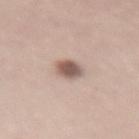workup: catalogued during a skin exam; not biopsied | lesion size: ≈3 mm | patient: female, in their mid- to late 30s | acquisition: 15 mm crop, total-body photography | automated lesion analysis: an area of roughly 5.5 mm² and a shape-asymmetry score of about 0.2 (0 = symmetric); about 15 CIELAB-L* units darker than the surrounding skin and a normalized lesion–skin contrast near 9.5 | site: the lower back.